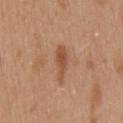Assessment:
The lesion was photographed on a routine skin check and not biopsied; there is no pathology result.
Context:
Imaged with white-light lighting. An algorithmic analysis of the crop reported roughly 9 lightness units darker than nearby skin and a lesion-to-skin contrast of about 7 (normalized; higher = more distinct). The software also gave a border-irregularity rating of about 4.5/10 and a color-variation rating of about 2/10. A region of skin cropped from a whole-body photographic capture, roughly 15 mm wide. The lesion is on the mid back. The patient is a female aged approximately 40.